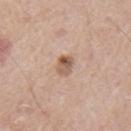The lesion was tiled from a total-body skin photograph and was not biopsied.
Longest diameter approximately 2.5 mm.
Located on the left upper arm.
A close-up tile cropped from a whole-body skin photograph, about 15 mm across.
Imaged with white-light lighting.
A male patient, aged 63 to 67.
The lesion-visualizer software estimated a lesion–skin lightness drop of about 12 and a normalized border contrast of about 8. The software also gave a nevus-likeness score of about 80/100 and a lesion-detection confidence of about 100/100.